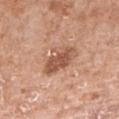imaging modality = 15 mm crop, total-body photography | diameter = about 5 mm | patient = female, aged around 75 | lighting = white-light illumination | automated metrics = an area of roughly 9.5 mm², an eccentricity of roughly 0.85, and a symmetry-axis asymmetry near 0.2; a lesion color around L≈54 a*≈23 b*≈31 in CIELAB, about 12 CIELAB-L* units darker than the surrounding skin, and a normalized lesion–skin contrast near 8; a border-irregularity rating of about 3/10 and a peripheral color-asymmetry measure near 1; a classifier nevus-likeness of about 30/100 | site = the right forearm.